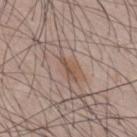No biopsy was performed on this lesion — it was imaged during a full skin examination and was not determined to be concerning. The patient is a male aged around 35. A region of skin cropped from a whole-body photographic capture, roughly 15 mm wide. Located on the mid back.